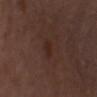Assessment: Recorded during total-body skin imaging; not selected for excision or biopsy. Clinical summary: A male patient, in their mid- to late 70s. The lesion is located on the chest. Measured at roughly 3 mm in maximum diameter. This is a white-light tile. A 15 mm close-up extracted from a 3D total-body photography capture. Automated image analysis of the tile measured a classifier nevus-likeness of about 10/100 and a lesion-detection confidence of about 100/100.